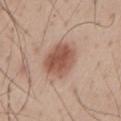A male subject, in their mid-40s. A 15 mm close-up extracted from a 3D total-body photography capture. From the front of the torso.